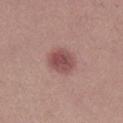tile lighting = white-light
image = 15 mm crop, total-body photography
location = the right lower leg
lesion size = ≈3.5 mm
subject = female, aged around 20